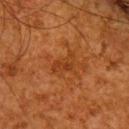Findings:
- follow-up: no biopsy performed (imaged during a skin exam)
- location: the back
- size: ≈3 mm
- tile lighting: cross-polarized
- image source: total-body-photography crop, ~15 mm field of view
- patient: male, approximately 65 years of age